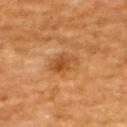Findings:
- site · the upper back
- subject · male, aged 58 to 62
- imaging modality · ~15 mm tile from a whole-body skin photo
- illumination · cross-polarized
- image-analysis metrics · a classifier nevus-likeness of about 40/100 and a lesion-detection confidence of about 100/100